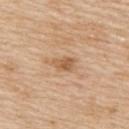Impression: Imaged during a routine full-body skin examination; the lesion was not biopsied and no histopathology is available. Clinical summary: The lesion is on the upper back. The subject is a male approximately 60 years of age. The total-body-photography lesion software estimated an automated nevus-likeness rating near 20 out of 100 and lesion-presence confidence of about 100/100. Cropped from a whole-body photographic skin survey; the tile spans about 15 mm. The tile uses white-light illumination. The recorded lesion diameter is about 2.5 mm.